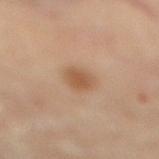<record>
<biopsy_status>not biopsied; imaged during a skin examination</biopsy_status>
<patient>
  <sex>female</sex>
  <age_approx>60</age_approx>
</patient>
<image>
  <source>total-body photography crop</source>
  <field_of_view_mm>15</field_of_view_mm>
</image>
<lighting>cross-polarized</lighting>
<site>leg</site>
<lesion_size>
  <long_diameter_mm_approx>3.0</long_diameter_mm_approx>
</lesion_size>
<automated_metrics>
  <area_mm2_approx>6.0</area_mm2_approx>
  <eccentricity>0.5</eccentricity>
  <shape_asymmetry>0.15</shape_asymmetry>
  <cielab_L>54</cielab_L>
  <cielab_a>19</cielab_a>
  <cielab_b>33</cielab_b>
  <vs_skin_contrast_norm>7.0</vs_skin_contrast_norm>
  <color_variation_0_10>2.0</color_variation_0_10>
  <peripheral_color_asymmetry>0.5</peripheral_color_asymmetry>
</automated_metrics>
</record>